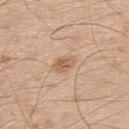Recorded during total-body skin imaging; not selected for excision or biopsy. Approximately 3 mm at its widest. Cropped from a total-body skin-imaging series; the visible field is about 15 mm. Automated tile analysis of the lesion measured a border-irregularity index near 2/10. The lesion is located on the upper back. The patient is a male aged approximately 50.